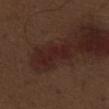Recorded during total-body skin imaging; not selected for excision or biopsy.
The tile uses white-light illumination.
A male patient aged around 70.
A lesion tile, about 15 mm wide, cut from a 3D total-body photograph.
Automated image analysis of the tile measured an area of roughly 6.5 mm², an eccentricity of roughly 0.95, and a symmetry-axis asymmetry near 0.4. And it measured border irregularity of about 6 on a 0–10 scale, a within-lesion color-variation index near 1.5/10, and radial color variation of about 0.5.
Located on the abdomen.